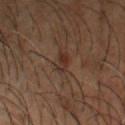{
  "biopsy_status": "not biopsied; imaged during a skin examination",
  "lesion_size": {
    "long_diameter_mm_approx": 3.0
  },
  "lighting": "cross-polarized",
  "automated_metrics": {
    "vs_skin_contrast_norm": 7.0
  },
  "site": "head or neck",
  "image": {
    "source": "total-body photography crop",
    "field_of_view_mm": 15
  },
  "patient": {
    "sex": "male",
    "age_approx": 50
  }
}